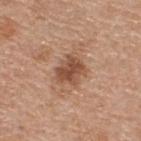Impression: Part of a total-body skin-imaging series; this lesion was reviewed on a skin check and was not flagged for biopsy. Acquisition and patient details: On the back. Longest diameter approximately 3.5 mm. Automated image analysis of the tile measured a lesion area of about 8 mm², a shape eccentricity near 0.55, and a symmetry-axis asymmetry near 0.25. The analysis additionally found a border-irregularity index near 3/10, a color-variation rating of about 3.5/10, and radial color variation of about 1. The software also gave an automated nevus-likeness rating near 5 out of 100 and a lesion-detection confidence of about 100/100. Cropped from a total-body skin-imaging series; the visible field is about 15 mm. A male patient, roughly 55 years of age.